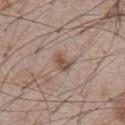workup — no biopsy performed (imaged during a skin exam); body site — the abdomen; patient — male, approximately 55 years of age; tile lighting — white-light; image source — ~15 mm tile from a whole-body skin photo.